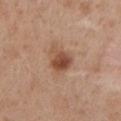workup = no biopsy performed (imaged during a skin exam) | imaging modality = total-body-photography crop, ~15 mm field of view | image-analysis metrics = border irregularity of about 3.5 on a 0–10 scale and a color-variation rating of about 5/10; a nevus-likeness score of about 90/100 and lesion-presence confidence of about 100/100 | lighting = white-light illumination | location = the arm | subject = female, aged 38 to 42 | diameter = ≈3.5 mm.